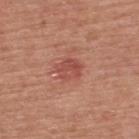Captured during whole-body skin photography for melanoma surveillance; the lesion was not biopsied. The lesion is on the upper back. Cropped from a whole-body photographic skin survey; the tile spans about 15 mm. Imaged with white-light lighting. A male patient, aged 53 to 57.